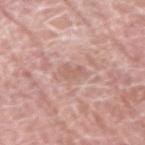biopsy status: catalogued during a skin exam; not biopsied
imaging modality: ~15 mm tile from a whole-body skin photo
patient: male, in their mid-70s
tile lighting: white-light
automated metrics: a lesion color around L≈61 a*≈21 b*≈26 in CIELAB, a lesion–skin lightness drop of about 7, and a lesion-to-skin contrast of about 5 (normalized; higher = more distinct); a border-irregularity index near 4.5/10, internal color variation of about 1.5 on a 0–10 scale, and a peripheral color-asymmetry measure near 0.5; a nevus-likeness score of about 0/100 and lesion-presence confidence of about 80/100
location: the right upper arm
lesion diameter: about 2.5 mm